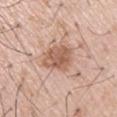Recorded during total-body skin imaging; not selected for excision or biopsy.
Imaged with white-light lighting.
Cropped from a total-body skin-imaging series; the visible field is about 15 mm.
A male subject, in their mid-50s.
Longest diameter approximately 4 mm.